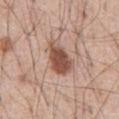notes: no biopsy performed (imaged during a skin exam)
image source: total-body-photography crop, ~15 mm field of view
subject: male, approximately 55 years of age
site: the front of the torso